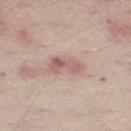Q: Was a biopsy performed?
A: imaged on a skin check; not biopsied
Q: How was this image acquired?
A: ~15 mm crop, total-body skin-cancer survey
Q: Patient demographics?
A: male, in their mid- to late 60s
Q: What lighting was used for the tile?
A: white-light illumination
Q: What did automated image analysis measure?
A: an average lesion color of about L≈60 a*≈19 b*≈22 (CIELAB), roughly 10 lightness units darker than nearby skin, and a normalized lesion–skin contrast near 7; border irregularity of about 3.5 on a 0–10 scale, a within-lesion color-variation index near 6.5/10, and radial color variation of about 2.5
Q: What is the anatomic site?
A: the left thigh